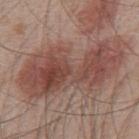Q: Was this lesion biopsied?
A: no biopsy performed (imaged during a skin exam)
Q: What is the imaging modality?
A: ~15 mm tile from a whole-body skin photo
Q: What lighting was used for the tile?
A: white-light
Q: What are the patient's age and sex?
A: male, aged 48 to 52
Q: Where on the body is the lesion?
A: the mid back